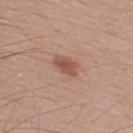Clinical impression: Recorded during total-body skin imaging; not selected for excision or biopsy. Image and clinical context: Captured under white-light illumination. The lesion-visualizer software estimated a footprint of about 5 mm², a shape eccentricity near 0.75, and two-axis asymmetry of about 0.15. And it measured a lesion color around L≈52 a*≈22 b*≈28 in CIELAB, about 10 CIELAB-L* units darker than the surrounding skin, and a normalized border contrast of about 7.5. The analysis additionally found border irregularity of about 1.5 on a 0–10 scale and internal color variation of about 2.5 on a 0–10 scale. It also reported a classifier nevus-likeness of about 90/100 and a detector confidence of about 100 out of 100 that the crop contains a lesion. On the upper back. This image is a 15 mm lesion crop taken from a total-body photograph. Approximately 3 mm at its widest. The subject is a male aged 38 to 42.